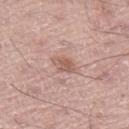Q: Is there a histopathology result?
A: no biopsy performed (imaged during a skin exam)
Q: How was the tile lit?
A: white-light
Q: Lesion location?
A: the left leg
Q: What are the patient's age and sex?
A: male, aged approximately 70
Q: How was this image acquired?
A: total-body-photography crop, ~15 mm field of view